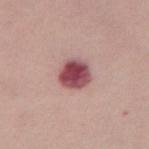No biopsy was performed on this lesion — it was imaged during a full skin examination and was not determined to be concerning.
This image is a 15 mm lesion crop taken from a total-body photograph.
A female subject roughly 45 years of age.
The lesion is on the right thigh.